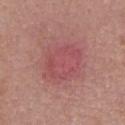The lesion was photographed on a routine skin check and not biopsied; there is no pathology result. A female subject roughly 50 years of age. The lesion is on the chest. A 15 mm close-up tile from a total-body photography series done for melanoma screening.